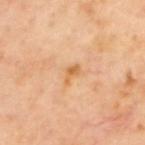Impression:
This lesion was catalogued during total-body skin photography and was not selected for biopsy.
Clinical summary:
A male subject, aged around 65. The total-body-photography lesion software estimated a mean CIELAB color near L≈64 a*≈22 b*≈43, a lesion–skin lightness drop of about 9, and a normalized border contrast of about 7. And it measured a border-irregularity index near 4/10, a color-variation rating of about 0.5/10, and a peripheral color-asymmetry measure near 0. Captured under cross-polarized illumination. The lesion's longest dimension is about 2.5 mm. Cropped from a total-body skin-imaging series; the visible field is about 15 mm. On the upper back.